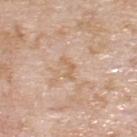Q: Was a biopsy performed?
A: no biopsy performed (imaged during a skin exam)
Q: What kind of image is this?
A: ~15 mm tile from a whole-body skin photo
Q: Where on the body is the lesion?
A: the upper back
Q: Patient demographics?
A: male, about 60 years old